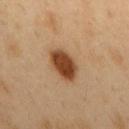No biopsy was performed on this lesion — it was imaged during a full skin examination and was not determined to be concerning. A roughly 15 mm field-of-view crop from a total-body skin photograph. Measured at roughly 4.5 mm in maximum diameter. From the mid back. Captured under cross-polarized illumination. A male subject, aged approximately 50.